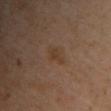A male patient roughly 50 years of age. An algorithmic analysis of the crop reported an area of roughly 3 mm² and an outline eccentricity of about 0.85 (0 = round, 1 = elongated). The software also gave border irregularity of about 4 on a 0–10 scale and radial color variation of about 0.5. It also reported a nevus-likeness score of about 5/100 and lesion-presence confidence of about 100/100. Measured at roughly 2.5 mm in maximum diameter. From the upper back. This image is a 15 mm lesion crop taken from a total-body photograph.